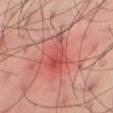imaging modality: 15 mm crop, total-body photography
subject: male, roughly 40 years of age
body site: the right thigh